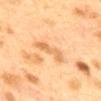workup: catalogued during a skin exam; not biopsied | acquisition: ~15 mm crop, total-body skin-cancer survey | image-analysis metrics: a mean CIELAB color near L≈58 a*≈19 b*≈38 and about 8 CIELAB-L* units darker than the surrounding skin | patient: female, aged 38 to 42 | size: about 4.5 mm | site: the mid back | lighting: cross-polarized.